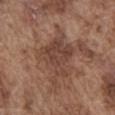{
  "biopsy_status": "not biopsied; imaged during a skin examination",
  "patient": {
    "sex": "male",
    "age_approx": 75
  },
  "image": {
    "source": "total-body photography crop",
    "field_of_view_mm": 15
  },
  "site": "abdomen"
}